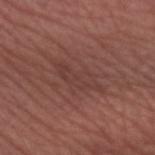{"biopsy_status": "not biopsied; imaged during a skin examination", "patient": {"sex": "female", "age_approx": 55}, "automated_metrics": {"border_irregularity_0_10": 5.0, "color_variation_0_10": 1.5, "nevus_likeness_0_100": 0, "lesion_detection_confidence_0_100": 65}, "image": {"source": "total-body photography crop", "field_of_view_mm": 15}, "lesion_size": {"long_diameter_mm_approx": 5.5}, "site": "left forearm", "lighting": "white-light"}